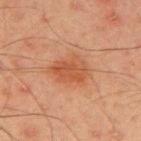Case summary:
* workup: no biopsy performed (imaged during a skin exam)
* anatomic site: the upper back
* image source: ~15 mm tile from a whole-body skin photo
* lighting: cross-polarized illumination
* automated lesion analysis: a mean CIELAB color near L≈44 a*≈24 b*≈32, roughly 7 lightness units darker than nearby skin, and a normalized border contrast of about 6.5
* patient: male, roughly 45 years of age
* size: ≈4 mm